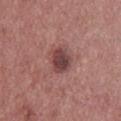The lesion is on the back. This image is a 15 mm lesion crop taken from a total-body photograph. The tile uses white-light illumination. Longest diameter approximately 3.5 mm. A male patient roughly 25 years of age.